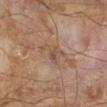Assessment: Imaged during a routine full-body skin examination; the lesion was not biopsied and no histopathology is available.